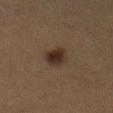Imaged during a routine full-body skin examination; the lesion was not biopsied and no histopathology is available.
A 15 mm close-up tile from a total-body photography series done for melanoma screening.
A male subject, aged around 50.
The lesion is on the abdomen.
This is a cross-polarized tile.